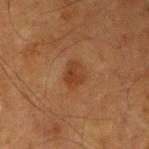Captured during whole-body skin photography for melanoma surveillance; the lesion was not biopsied.
Captured under cross-polarized illumination.
From the left forearm.
A male subject, aged around 75.
A roughly 15 mm field-of-view crop from a total-body skin photograph.